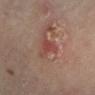Background: This is a cross-polarized tile. Located on the leg. A roughly 15 mm field-of-view crop from a total-body skin photograph. The recorded lesion diameter is about 4 mm. The subject is a female in their mid-30s.